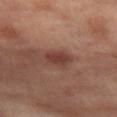Q: Was a biopsy performed?
A: imaged on a skin check; not biopsied
Q: How was the tile lit?
A: cross-polarized illumination
Q: What is the lesion's diameter?
A: ≈3 mm
Q: What is the imaging modality?
A: 15 mm crop, total-body photography
Q: Lesion location?
A: the left thigh
Q: What are the patient's age and sex?
A: female, aged 53 to 57